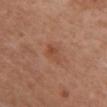| feature | finding |
|---|---|
| biopsy status | no biopsy performed (imaged during a skin exam) |
| body site | the front of the torso |
| diameter | ≈2.5 mm |
| acquisition | 15 mm crop, total-body photography |
| automated metrics | an area of roughly 4 mm², an outline eccentricity of about 0.75 (0 = round, 1 = elongated), and two-axis asymmetry of about 0.3; a color-variation rating of about 3.5/10 and a peripheral color-asymmetry measure near 1.5; a nevus-likeness score of about 5/100 and a lesion-detection confidence of about 100/100 |
| patient | female, aged around 70 |
| lighting | white-light illumination |